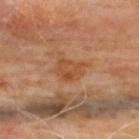Impression:
The lesion was tiled from a total-body skin photograph and was not biopsied.
Image and clinical context:
Cropped from a total-body skin-imaging series; the visible field is about 15 mm. On the upper back. Automated image analysis of the tile measured a mean CIELAB color near L≈47 a*≈23 b*≈36 and about 7 CIELAB-L* units darker than the surrounding skin. The software also gave an automated nevus-likeness rating near 0 out of 100. The patient is a male in their mid-60s.